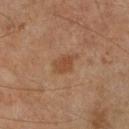This lesion was catalogued during total-body skin photography and was not selected for biopsy. A 15 mm crop from a total-body photograph taken for skin-cancer surveillance. On the right lower leg. A male subject, in their mid- to late 60s. The tile uses cross-polarized illumination.